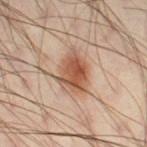follow-up = no biopsy performed (imaged during a skin exam); patient = male, aged 38 to 42; diameter = about 5 mm; acquisition = ~15 mm tile from a whole-body skin photo; location = the right thigh; lighting = cross-polarized; TBP lesion metrics = internal color variation of about 6 on a 0–10 scale.